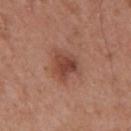Impression:
Imaged during a routine full-body skin examination; the lesion was not biopsied and no histopathology is available.
Image and clinical context:
Cropped from a total-body skin-imaging series; the visible field is about 15 mm. Automated tile analysis of the lesion measured a border-irregularity rating of about 2.5/10, a color-variation rating of about 4.5/10, and peripheral color asymmetry of about 1.5. A male patient, in their mid-50s. Measured at roughly 3.5 mm in maximum diameter. From the mid back. Captured under white-light illumination.